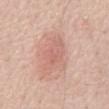| feature | finding |
|---|---|
| biopsy status | total-body-photography surveillance lesion; no biopsy |
| patient | male, approximately 70 years of age |
| acquisition | ~15 mm crop, total-body skin-cancer survey |
| location | the abdomen |